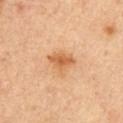patient: male, in their mid- to late 50s; illumination: cross-polarized; diameter: about 3 mm; image source: ~15 mm crop, total-body skin-cancer survey; anatomic site: the left upper arm.